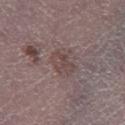Imaged during a routine full-body skin examination; the lesion was not biopsied and no histopathology is available. On the right lower leg. The subject is a male aged approximately 65. The tile uses white-light illumination. A roughly 15 mm field-of-view crop from a total-body skin photograph.